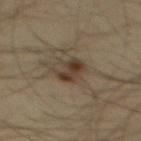follow-up = total-body-photography surveillance lesion; no biopsy | subject = male, in their mid-30s | lighting = cross-polarized | image = ~15 mm tile from a whole-body skin photo | automated metrics = a lesion area of about 6.5 mm², a shape eccentricity near 0.75, and a symmetry-axis asymmetry near 0.25; an average lesion color of about L≈32 a*≈11 b*≈23 (CIELAB), a lesion–skin lightness drop of about 9, and a normalized lesion–skin contrast near 8.5; border irregularity of about 3 on a 0–10 scale, internal color variation of about 5.5 on a 0–10 scale, and peripheral color asymmetry of about 1.5; a classifier nevus-likeness of about 70/100 | site = the back.